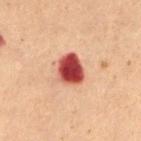| feature | finding |
|---|---|
| biopsy status | no biopsy performed (imaged during a skin exam) |
| body site | the mid back |
| image | ~15 mm crop, total-body skin-cancer survey |
| patient | female, aged 78 to 82 |
| lighting | cross-polarized illumination |
| diameter | about 3.5 mm |